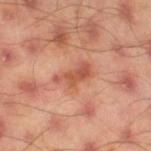| key | value |
|---|---|
| biopsy status | no biopsy performed (imaged during a skin exam) |
| subject | male, aged approximately 45 |
| image | ~15 mm crop, total-body skin-cancer survey |
| location | the right thigh |